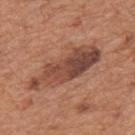Impression: The lesion was photographed on a routine skin check and not biopsied; there is no pathology result. Context: About 9.5 mm across. Automated tile analysis of the lesion measured an outline eccentricity of about 0.95 (0 = round, 1 = elongated) and two-axis asymmetry of about 0.25. The software also gave a mean CIELAB color near L≈46 a*≈23 b*≈28 and a normalized border contrast of about 9. It also reported a border-irregularity rating of about 4/10, a within-lesion color-variation index near 7.5/10, and radial color variation of about 2.5. It also reported an automated nevus-likeness rating near 20 out of 100. On the back. Imaged with white-light lighting. Cropped from a whole-body photographic skin survey; the tile spans about 15 mm. A male patient aged 63 to 67.